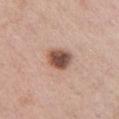tile lighting: white-light illumination
image-analysis metrics: a within-lesion color-variation index near 5.5/10 and radial color variation of about 1.5
body site: the front of the torso
size: ~3 mm (longest diameter)
imaging modality: 15 mm crop, total-body photography
patient: female, aged 38–42